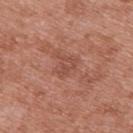biopsy status: catalogued during a skin exam; not biopsied
patient: female, about 40 years old
site: the upper back
image: ~15 mm tile from a whole-body skin photo